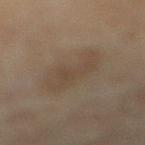Q: Was this lesion biopsied?
A: catalogued during a skin exam; not biopsied
Q: What is the anatomic site?
A: the right lower leg
Q: Automated lesion metrics?
A: a border-irregularity index near 4/10
Q: What is the lesion's diameter?
A: ≈6.5 mm
Q: What is the imaging modality?
A: ~15 mm tile from a whole-body skin photo
Q: What are the patient's age and sex?
A: female, aged around 60